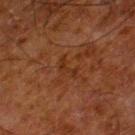biopsy_status: not biopsied; imaged during a skin examination
image:
  source: total-body photography crop
  field_of_view_mm: 15
site: left thigh
lighting: cross-polarized
lesion_size:
  long_diameter_mm_approx: 2.5
patient:
  sex: male
  age_approx: 80